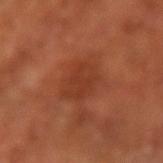Assessment:
Part of a total-body skin-imaging series; this lesion was reviewed on a skin check and was not flagged for biopsy.
Clinical summary:
Automated tile analysis of the lesion measured border irregularity of about 2 on a 0–10 scale and a peripheral color-asymmetry measure near 0.5. The recorded lesion diameter is about 3.5 mm. A lesion tile, about 15 mm wide, cut from a 3D total-body photograph. From the left arm. A male patient, approximately 65 years of age. Captured under cross-polarized illumination.